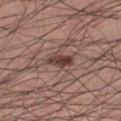image source — 15 mm crop, total-body photography
subject — male, in their mid- to late 20s
lesion diameter — ~3 mm (longest diameter)
illumination — white-light
body site — the right thigh
image-analysis metrics — a footprint of about 5 mm², an outline eccentricity of about 0.8 (0 = round, 1 = elongated), and two-axis asymmetry of about 0.3; a border-irregularity index near 3/10, a color-variation rating of about 5/10, and a peripheral color-asymmetry measure near 2; a lesion-detection confidence of about 100/100